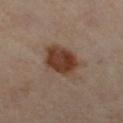Part of a total-body skin-imaging series; this lesion was reviewed on a skin check and was not flagged for biopsy.